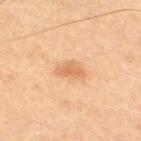A 15 mm close-up tile from a total-body photography series done for melanoma screening. On the right thigh. A male subject in their mid-60s. Approximately 3 mm at its widest.